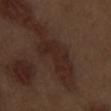| field | value |
|---|---|
| follow-up | no biopsy performed (imaged during a skin exam) |
| lighting | white-light illumination |
| site | the left upper arm |
| subject | male, about 70 years old |
| size | ≈4 mm |
| imaging modality | total-body-photography crop, ~15 mm field of view |